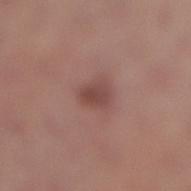Clinical impression:
The lesion was tiled from a total-body skin photograph and was not biopsied.
Context:
A female patient aged 18 to 22. About 3 mm across. A close-up tile cropped from a whole-body skin photograph, about 15 mm across. On the right lower leg. Imaged with white-light lighting.